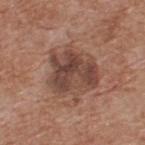A close-up tile cropped from a whole-body skin photograph, about 15 mm across. The subject is a male aged approximately 60. Captured under white-light illumination. The lesion is located on the upper back.